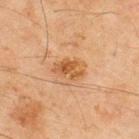Clinical impression: No biopsy was performed on this lesion — it was imaged during a full skin examination and was not determined to be concerning. Image and clinical context: A male subject, aged 43 to 47. Located on the upper back. Cropped from a whole-body photographic skin survey; the tile spans about 15 mm.